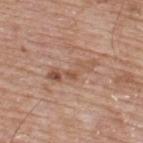Clinical impression:
Recorded during total-body skin imaging; not selected for excision or biopsy.
Context:
A male subject about 65 years old. A roughly 15 mm field-of-view crop from a total-body skin photograph. On the upper back.